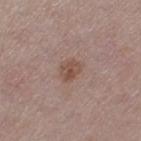| field | value |
|---|---|
| notes | no biopsy performed (imaged during a skin exam) |
| image source | ~15 mm tile from a whole-body skin photo |
| illumination | white-light |
| automated lesion analysis | an average lesion color of about L≈49 a*≈18 b*≈25 (CIELAB), about 8 CIELAB-L* units darker than the surrounding skin, and a normalized border contrast of about 7 |
| subject | female, approximately 30 years of age |
| site | the left thigh |